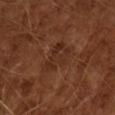Impression:
This lesion was catalogued during total-body skin photography and was not selected for biopsy.
Acquisition and patient details:
A 15 mm close-up tile from a total-body photography series done for melanoma screening. Approximately 4.5 mm at its widest. Imaged with cross-polarized lighting. Automated image analysis of the tile measured an outline eccentricity of about 0.85 (0 = round, 1 = elongated) and a shape-asymmetry score of about 0.4 (0 = symmetric). A male patient roughly 65 years of age.